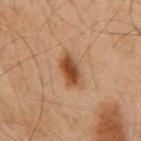The lesion was photographed on a routine skin check and not biopsied; there is no pathology result.
A male patient approximately 65 years of age.
Measured at roughly 4 mm in maximum diameter.
The lesion-visualizer software estimated a lesion area of about 6.5 mm² and two-axis asymmetry of about 0.2. It also reported a border-irregularity index near 2/10, a within-lesion color-variation index near 4.5/10, and a peripheral color-asymmetry measure near 1.5. The analysis additionally found a classifier nevus-likeness of about 100/100 and a detector confidence of about 100 out of 100 that the crop contains a lesion.
This is a cross-polarized tile.
On the mid back.
A 15 mm close-up extracted from a 3D total-body photography capture.